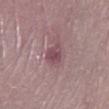follow-up: total-body-photography surveillance lesion; no biopsy | size: about 2.5 mm | lighting: white-light illumination | automated metrics: a lesion color around L≈47 a*≈23 b*≈13 in CIELAB and a lesion–skin lightness drop of about 9; a border-irregularity index near 2/10, internal color variation of about 2.5 on a 0–10 scale, and a peripheral color-asymmetry measure near 1; a detector confidence of about 90 out of 100 that the crop contains a lesion | subject: female, aged around 70 | site: the left lower leg | image source: total-body-photography crop, ~15 mm field of view.